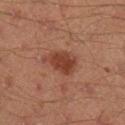Recorded during total-body skin imaging; not selected for excision or biopsy.
The tile uses cross-polarized illumination.
An algorithmic analysis of the crop reported a footprint of about 8 mm², an outline eccentricity of about 0.75 (0 = round, 1 = elongated), and a symmetry-axis asymmetry near 0.3. And it measured a mean CIELAB color near L≈33 a*≈21 b*≈25. The analysis additionally found border irregularity of about 3 on a 0–10 scale, internal color variation of about 2.5 on a 0–10 scale, and a peripheral color-asymmetry measure near 1. The software also gave a classifier nevus-likeness of about 95/100.
Located on the right lower leg.
A male subject approximately 45 years of age.
A lesion tile, about 15 mm wide, cut from a 3D total-body photograph.